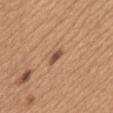Clinical impression: This lesion was catalogued during total-body skin photography and was not selected for biopsy. Acquisition and patient details: The patient is a female roughly 40 years of age. On the mid back. Imaged with white-light lighting. The lesion-visualizer software estimated an average lesion color of about L≈51 a*≈22 b*≈31 (CIELAB), about 12 CIELAB-L* units darker than the surrounding skin, and a normalized border contrast of about 8.5. And it measured a border-irregularity rating of about 3/10, a color-variation rating of about 0/10, and a peripheral color-asymmetry measure near 0. The analysis additionally found a nevus-likeness score of about 65/100 and a detector confidence of about 100 out of 100 that the crop contains a lesion. Approximately 2.5 mm at its widest. Cropped from a whole-body photographic skin survey; the tile spans about 15 mm.